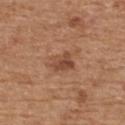  biopsy_status: not biopsied; imaged during a skin examination
  lighting: white-light
  site: upper back
  automated_metrics:
    cielab_L: 47
    cielab_a: 22
    cielab_b: 31
    vs_skin_darker_L: 10.0
    vs_skin_contrast_norm: 7.0
    border_irregularity_0_10: 3.0
    color_variation_0_10: 5.0
    peripheral_color_asymmetry: 2.0
    nevus_likeness_0_100: 75
    lesion_detection_confidence_0_100: 100
  lesion_size:
    long_diameter_mm_approx: 3.0
  image:
    source: total-body photography crop
    field_of_view_mm: 15
  patient:
    sex: male
    age_approx: 70